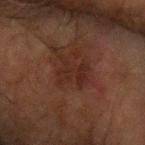| key | value |
|---|---|
| biopsy status | no biopsy performed (imaged during a skin exam) |
| image source | 15 mm crop, total-body photography |
| location | the right forearm |
| automated lesion analysis | a footprint of about 7 mm², a shape eccentricity near 0.5, and two-axis asymmetry of about 0.35; a classifier nevus-likeness of about 0/100 and lesion-presence confidence of about 100/100 |
| lighting | cross-polarized |
| patient | male, aged 63–67 |